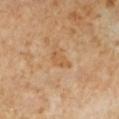{
  "biopsy_status": "not biopsied; imaged during a skin examination",
  "site": "left lower leg",
  "image": {
    "source": "total-body photography crop",
    "field_of_view_mm": 15
  },
  "lesion_size": {
    "long_diameter_mm_approx": 3.0
  },
  "patient": {
    "sex": "female",
    "age_approx": 50
  }
}